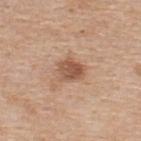The lesion was photographed on a routine skin check and not biopsied; there is no pathology result. This image is a 15 mm lesion crop taken from a total-body photograph. A male subject, aged around 60. On the upper back.